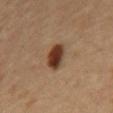The total-body-photography lesion software estimated a mean CIELAB color near L≈34 a*≈20 b*≈28, about 16 CIELAB-L* units darker than the surrounding skin, and a normalized lesion–skin contrast near 13.5. The analysis additionally found a border-irregularity rating of about 2/10, a color-variation rating of about 4/10, and radial color variation of about 1. From the chest. Measured at roughly 3.5 mm in maximum diameter. A 15 mm close-up extracted from a 3D total-body photography capture. The tile uses cross-polarized illumination. A male patient approximately 65 years of age.